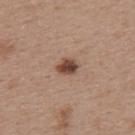Clinical impression: Captured during whole-body skin photography for melanoma surveillance; the lesion was not biopsied. Clinical summary: Cropped from a whole-body photographic skin survey; the tile spans about 15 mm. Located on the upper back. A female patient aged 38 to 42. The total-body-photography lesion software estimated an outline eccentricity of about 0.65 (0 = round, 1 = elongated) and a symmetry-axis asymmetry near 0.25. The analysis additionally found a border-irregularity index near 2/10, a within-lesion color-variation index near 3.5/10, and radial color variation of about 1. It also reported a nevus-likeness score of about 95/100 and a detector confidence of about 100 out of 100 that the crop contains a lesion. The recorded lesion diameter is about 2.5 mm.